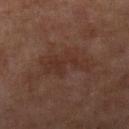image:
  source: total-body photography crop
  field_of_view_mm: 15
patient:
  sex: female
  age_approx: 60
site: right lower leg
lighting: cross-polarized
lesion_size:
  long_diameter_mm_approx: 6.0